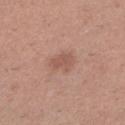Assessment:
This lesion was catalogued during total-body skin photography and was not selected for biopsy.
Image and clinical context:
On the left lower leg. A female patient, roughly 30 years of age. A lesion tile, about 15 mm wide, cut from a 3D total-body photograph.